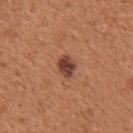notes = no biopsy performed (imaged during a skin exam); diameter = ~2.5 mm (longest diameter); automated metrics = a mean CIELAB color near L≈42 a*≈24 b*≈28, about 14 CIELAB-L* units darker than the surrounding skin, and a normalized lesion–skin contrast near 11; image = ~15 mm tile from a whole-body skin photo; subject = male, aged approximately 55; anatomic site = the mid back; tile lighting = white-light.